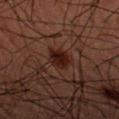{"biopsy_status": "not biopsied; imaged during a skin examination", "image": {"source": "total-body photography crop", "field_of_view_mm": 15}, "lesion_size": {"long_diameter_mm_approx": 3.0}, "site": "left forearm", "lighting": "cross-polarized", "patient": {"sex": "male", "age_approx": 60}}